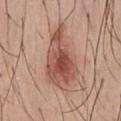Impression:
No biopsy was performed on this lesion — it was imaged during a full skin examination and was not determined to be concerning.
Context:
Approximately 8 mm at its widest. Captured under white-light illumination. A 15 mm close-up tile from a total-body photography series done for melanoma screening. On the chest. The subject is a male aged 28–32.